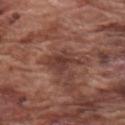biopsy status: total-body-photography surveillance lesion; no biopsy
size: ~4 mm (longest diameter)
subject: male, approximately 75 years of age
anatomic site: the right upper arm
image-analysis metrics: an average lesion color of about L≈38 a*≈22 b*≈24 (CIELAB), about 9 CIELAB-L* units darker than the surrounding skin, and a normalized lesion–skin contrast near 8
image: total-body-photography crop, ~15 mm field of view
illumination: white-light illumination